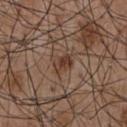Cropped from a whole-body photographic skin survey; the tile spans about 15 mm.
A male subject in their mid-50s.
Automated image analysis of the tile measured a mean CIELAB color near L≈38 a*≈18 b*≈26 and a lesion–skin lightness drop of about 9. The analysis additionally found an automated nevus-likeness rating near 30 out of 100.
Longest diameter approximately 2.5 mm.
Located on the chest.